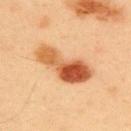Clinical impression: Part of a total-body skin-imaging series; this lesion was reviewed on a skin check and was not flagged for biopsy. Image and clinical context: A male patient, aged around 40. Located on the upper back. A 15 mm crop from a total-body photograph taken for skin-cancer surveillance. About 7.5 mm across.